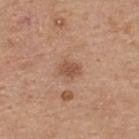Captured during whole-body skin photography for melanoma surveillance; the lesion was not biopsied. The total-body-photography lesion software estimated an area of roughly 3.5 mm². The analysis additionally found a mean CIELAB color near L≈52 a*≈21 b*≈31, roughly 10 lightness units darker than nearby skin, and a normalized lesion–skin contrast near 7. And it measured border irregularity of about 2 on a 0–10 scale, a color-variation rating of about 1.5/10, and a peripheral color-asymmetry measure near 0.5. And it measured an automated nevus-likeness rating near 10 out of 100 and a detector confidence of about 100 out of 100 that the crop contains a lesion. The tile uses white-light illumination. A 15 mm crop from a total-body photograph taken for skin-cancer surveillance. A male subject, aged approximately 65. On the back. The recorded lesion diameter is about 2.5 mm.